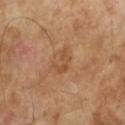Image and clinical context: An algorithmic analysis of the crop reported a footprint of about 4 mm² and a shape eccentricity near 0.7. It also reported border irregularity of about 6 on a 0–10 scale, internal color variation of about 0 on a 0–10 scale, and a peripheral color-asymmetry measure near 0. It also reported a nevus-likeness score of about 0/100 and a detector confidence of about 100 out of 100 that the crop contains a lesion. A male subject aged 63–67. This image is a 15 mm lesion crop taken from a total-body photograph.